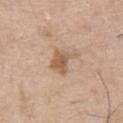Case summary:
- follow-up: no biopsy performed (imaged during a skin exam)
- subject: male, roughly 75 years of age
- body site: the upper back
- lighting: white-light illumination
- lesion diameter: ~4 mm (longest diameter)
- acquisition: ~15 mm crop, total-body skin-cancer survey